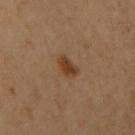Impression:
Recorded during total-body skin imaging; not selected for excision or biopsy.
Clinical summary:
A female patient aged 58 to 62. The lesion is on the upper back. The tile uses cross-polarized illumination. A close-up tile cropped from a whole-body skin photograph, about 15 mm across. An algorithmic analysis of the crop reported roughly 9 lightness units darker than nearby skin and a normalized lesion–skin contrast near 9. The recorded lesion diameter is about 3 mm.